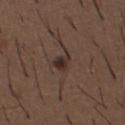Captured during whole-body skin photography for melanoma surveillance; the lesion was not biopsied. A 15 mm close-up extracted from a 3D total-body photography capture. The total-body-photography lesion software estimated a footprint of about 3.5 mm² and an outline eccentricity of about 0.7 (0 = round, 1 = elongated). The analysis additionally found a lesion color around L≈29 a*≈15 b*≈20 in CIELAB and a lesion-to-skin contrast of about 9.5 (normalized; higher = more distinct). The analysis additionally found a nevus-likeness score of about 95/100 and lesion-presence confidence of about 100/100. On the chest. The tile uses white-light illumination. The subject is a male aged around 50. Longest diameter approximately 2.5 mm.